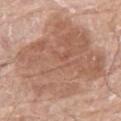Captured during whole-body skin photography for melanoma surveillance; the lesion was not biopsied.
The lesion's longest dimension is about 11 mm.
The total-body-photography lesion software estimated peripheral color asymmetry of about 2.
This is a white-light tile.
A lesion tile, about 15 mm wide, cut from a 3D total-body photograph.
A male subject, in their 80s.
Located on the right thigh.